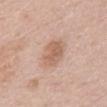The lesion was photographed on a routine skin check and not biopsied; there is no pathology result.
Located on the chest.
Automated image analysis of the tile measured a lesion area of about 9 mm². The software also gave a mean CIELAB color near L≈61 a*≈19 b*≈29 and a normalized lesion–skin contrast near 6.5.
Imaged with white-light lighting.
A close-up tile cropped from a whole-body skin photograph, about 15 mm across.
A male subject, roughly 60 years of age.